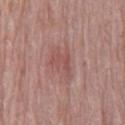Captured under white-light illumination.
Located on the lower back.
The subject is a male aged 68 to 72.
A close-up tile cropped from a whole-body skin photograph, about 15 mm across.